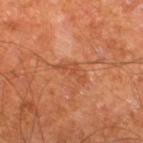Findings:
• workup — total-body-photography surveillance lesion; no biopsy
• site — the leg
• subject — male, aged 63–67
• image — 15 mm crop, total-body photography